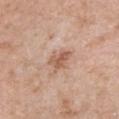* biopsy status: catalogued during a skin exam; not biopsied
* imaging modality: ~15 mm crop, total-body skin-cancer survey
* patient: female, in their 40s
* body site: the chest